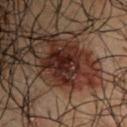- biopsy status · catalogued during a skin exam; not biopsied
- lighting · cross-polarized
- imaging modality · total-body-photography crop, ~15 mm field of view
- location · the left upper arm
- TBP lesion metrics · border irregularity of about 4 on a 0–10 scale, internal color variation of about 4.5 on a 0–10 scale, and peripheral color asymmetry of about 1.5; a classifier nevus-likeness of about 0/100 and lesion-presence confidence of about 100/100
- patient · male, aged approximately 50